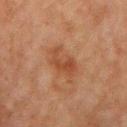Clinical impression: The lesion was tiled from a total-body skin photograph and was not biopsied. Background: The lesion's longest dimension is about 5 mm. The subject is a male aged 78–82. A 15 mm close-up extracted from a 3D total-body photography capture. An algorithmic analysis of the crop reported an eccentricity of roughly 0.85. The software also gave a lesion color around L≈37 a*≈20 b*≈28 in CIELAB and a normalized border contrast of about 6.5. The software also gave a border-irregularity index near 6/10, a within-lesion color-variation index near 3/10, and peripheral color asymmetry of about 1. The software also gave a classifier nevus-likeness of about 5/100. The tile uses cross-polarized illumination. On the mid back.